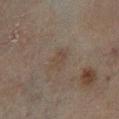workup: catalogued during a skin exam; not biopsied | size: about 2.5 mm | illumination: cross-polarized illumination | image: total-body-photography crop, ~15 mm field of view | subject: male, aged around 70 | body site: the left lower leg.